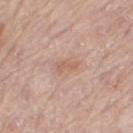workup: no biopsy performed (imaged during a skin exam); subject: female, approximately 65 years of age; diameter: ≈3 mm; image: ~15 mm tile from a whole-body skin photo; tile lighting: white-light; location: the left thigh.